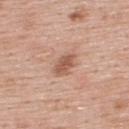Imaged during a routine full-body skin examination; the lesion was not biopsied and no histopathology is available.
Automated image analysis of the tile measured a lesion area of about 5 mm² and two-axis asymmetry of about 0.25.
A male subject, approximately 55 years of age.
Located on the upper back.
Longest diameter approximately 3 mm.
Captured under white-light illumination.
A region of skin cropped from a whole-body photographic capture, roughly 15 mm wide.